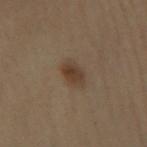Captured during whole-body skin photography for melanoma surveillance; the lesion was not biopsied.
Automated tile analysis of the lesion measured a mean CIELAB color near L≈32 a*≈12 b*≈23, a lesion–skin lightness drop of about 7, and a normalized border contrast of about 7.5. It also reported border irregularity of about 1.5 on a 0–10 scale, a within-lesion color-variation index near 2.5/10, and peripheral color asymmetry of about 1. It also reported a classifier nevus-likeness of about 95/100 and a lesion-detection confidence of about 100/100.
Captured under cross-polarized illumination.
From the right lower leg.
A female subject, about 50 years old.
Approximately 3 mm at its widest.
Cropped from a whole-body photographic skin survey; the tile spans about 15 mm.